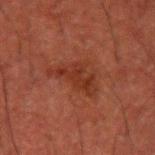patient: male, aged approximately 50
image: ~15 mm crop, total-body skin-cancer survey
location: the left upper arm